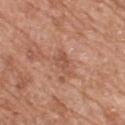Recorded during total-body skin imaging; not selected for excision or biopsy. Cropped from a whole-body photographic skin survey; the tile spans about 15 mm. A male patient, in their 60s. Located on the upper back.